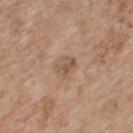– workup — no biopsy performed (imaged during a skin exam)
– body site — the back
– subject — male, aged 68 to 72
– image source — 15 mm crop, total-body photography